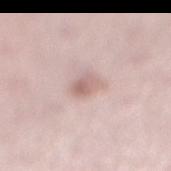The lesion was tiled from a total-body skin photograph and was not biopsied. A female patient, aged approximately 35. The tile uses white-light illumination. An algorithmic analysis of the crop reported peripheral color asymmetry of about 2. A roughly 15 mm field-of-view crop from a total-body skin photograph. The lesion's longest dimension is about 2.5 mm. The lesion is on the right lower leg.